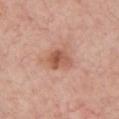Clinical impression:
Imaged during a routine full-body skin examination; the lesion was not biopsied and no histopathology is available.
Background:
The lesion is on the chest. A male subject, in their 60s. Automated image analysis of the tile measured a footprint of about 6 mm² and a symmetry-axis asymmetry near 0.25. It also reported a lesion–skin lightness drop of about 11 and a lesion-to-skin contrast of about 8 (normalized; higher = more distinct). The analysis additionally found a border-irregularity index near 2.5/10, a color-variation rating of about 6/10, and a peripheral color-asymmetry measure near 2. This is a white-light tile. A close-up tile cropped from a whole-body skin photograph, about 15 mm across. Approximately 3 mm at its widest.